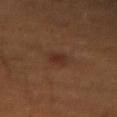Imaged during a routine full-body skin examination; the lesion was not biopsied and no histopathology is available.
The lesion is located on the left forearm.
The patient is a male in their 60s.
A region of skin cropped from a whole-body photographic capture, roughly 15 mm wide.
Measured at roughly 2.5 mm in maximum diameter.
An algorithmic analysis of the crop reported an area of roughly 3.5 mm² and two-axis asymmetry of about 0.25. The analysis additionally found a mean CIELAB color near L≈27 a*≈18 b*≈24, roughly 6 lightness units darker than nearby skin, and a lesion-to-skin contrast of about 6.5 (normalized; higher = more distinct). It also reported border irregularity of about 2.5 on a 0–10 scale and a peripheral color-asymmetry measure near 0.5.
Captured under cross-polarized illumination.